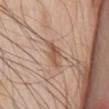{"biopsy_status": "not biopsied; imaged during a skin examination", "lesion_size": {"long_diameter_mm_approx": 3.5}, "patient": {"sex": "male", "age_approx": 60}, "automated_metrics": {"area_mm2_approx": 4.5, "eccentricity": 0.8, "shape_asymmetry": 0.3, "border_irregularity_0_10": 4.0, "color_variation_0_10": 3.0, "lesion_detection_confidence_0_100": 100}, "site": "chest", "image": {"source": "total-body photography crop", "field_of_view_mm": 15}}